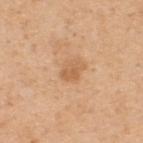The lesion was photographed on a routine skin check and not biopsied; there is no pathology result. The tile uses white-light illumination. A roughly 15 mm field-of-view crop from a total-body skin photograph. Measured at roughly 2.5 mm in maximum diameter. A male subject aged around 50. The lesion is located on the upper back.